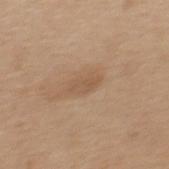  biopsy_status: not biopsied; imaged during a skin examination
  lighting: white-light
  lesion_size:
    long_diameter_mm_approx: 3.0
  site: back
  patient:
    sex: female
    age_approx: 40
  image:
    source: total-body photography crop
    field_of_view_mm: 15